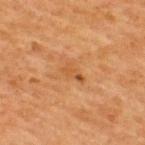Clinical impression:
This lesion was catalogued during total-body skin photography and was not selected for biopsy.
Clinical summary:
The lesion is located on the upper back. The total-body-photography lesion software estimated border irregularity of about 4 on a 0–10 scale, a color-variation rating of about 1/10, and peripheral color asymmetry of about 0. And it measured a classifier nevus-likeness of about 0/100 and a lesion-detection confidence of about 100/100. A female patient, in their 40s. A lesion tile, about 15 mm wide, cut from a 3D total-body photograph.